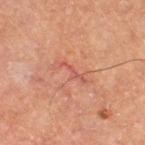- follow-up · total-body-photography surveillance lesion; no biopsy
- lesion diameter · ≈4 mm
- site · the right thigh
- illumination · cross-polarized
- image source · ~15 mm crop, total-body skin-cancer survey
- automated lesion analysis · a lesion area of about 3.5 mm² and an outline eccentricity of about 0.95 (0 = round, 1 = elongated); a mean CIELAB color near L≈45 a*≈25 b*≈25, a lesion–skin lightness drop of about 6, and a normalized border contrast of about 5; a border-irregularity rating of about 8/10 and a within-lesion color-variation index near 0/10; a classifier nevus-likeness of about 0/100 and lesion-presence confidence of about 95/100
- patient · female, approximately 60 years of age